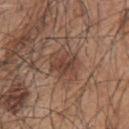Clinical impression: Recorded during total-body skin imaging; not selected for excision or biopsy. Image and clinical context: The lesion is located on the back. A male subject approximately 45 years of age. The tile uses white-light illumination. The total-body-photography lesion software estimated a border-irregularity rating of about 3.5/10, a within-lesion color-variation index near 5/10, and a peripheral color-asymmetry measure near 2. It also reported a nevus-likeness score of about 5/100 and a detector confidence of about 100 out of 100 that the crop contains a lesion. Longest diameter approximately 3.5 mm. A 15 mm close-up tile from a total-body photography series done for melanoma screening.